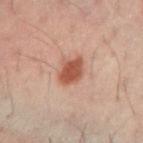notes = catalogued during a skin exam; not biopsied
automated metrics = a lesion area of about 7 mm², a shape eccentricity near 0.55, and a symmetry-axis asymmetry near 0.2; roughly 13 lightness units darker than nearby skin; a border-irregularity index near 2/10, a color-variation rating of about 2/10, and radial color variation of about 0.5
diameter = ≈3 mm
body site = the right forearm
tile lighting = cross-polarized
patient = male, aged approximately 40
acquisition = 15 mm crop, total-body photography